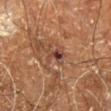Impression: Part of a total-body skin-imaging series; this lesion was reviewed on a skin check and was not flagged for biopsy. Context: The lesion is located on the right leg. A male subject, approximately 60 years of age. Captured under cross-polarized illumination. An algorithmic analysis of the crop reported a lesion area of about 3.5 mm². Cropped from a whole-body photographic skin survey; the tile spans about 15 mm. The lesion's longest dimension is about 3 mm.